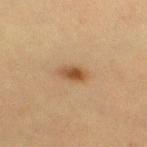Clinical impression:
The lesion was tiled from a total-body skin photograph and was not biopsied.
Image and clinical context:
A female patient aged 53 to 57. Cropped from a whole-body photographic skin survey; the tile spans about 15 mm. The lesion's longest dimension is about 3 mm. From the right thigh. The tile uses cross-polarized illumination.